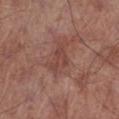This lesion was catalogued during total-body skin photography and was not selected for biopsy. A 15 mm close-up extracted from a 3D total-body photography capture. A male patient in their 70s. Located on the leg.